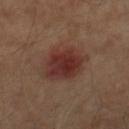Assessment: No biopsy was performed on this lesion — it was imaged during a full skin examination and was not determined to be concerning. Background: The lesion is on the right thigh. The subject is a male aged 53–57. A region of skin cropped from a whole-body photographic capture, roughly 15 mm wide.